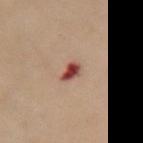No biopsy was performed on this lesion — it was imaged during a full skin examination and was not determined to be concerning. The total-body-photography lesion software estimated border irregularity of about 3 on a 0–10 scale, a color-variation rating of about 4.5/10, and radial color variation of about 1.5. The analysis additionally found a classifier nevus-likeness of about 0/100 and lesion-presence confidence of about 100/100. A female subject, aged 48 to 52. Longest diameter approximately 2.5 mm. The tile uses cross-polarized illumination. The lesion is on the chest. Cropped from a total-body skin-imaging series; the visible field is about 15 mm.